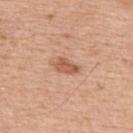• anatomic site · the upper back
• subject · male, aged 53–57
• size · ≈3 mm
• image · ~15 mm tile from a whole-body skin photo
• tile lighting · white-light illumination